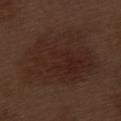A male patient, about 70 years old. About 10.5 mm across. The tile uses white-light illumination. The total-body-photography lesion software estimated roughly 5 lightness units darker than nearby skin and a normalized border contrast of about 6. The software also gave a border-irregularity index near 3/10, a color-variation rating of about 3.5/10, and peripheral color asymmetry of about 1. Located on the left thigh. Cropped from a whole-body photographic skin survey; the tile spans about 15 mm.